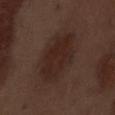  biopsy_status: not biopsied; imaged during a skin examination
  patient:
    sex: male
    age_approx: 70
  image:
    source: total-body photography crop
    field_of_view_mm: 15
  site: abdomen